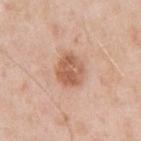Recorded during total-body skin imaging; not selected for excision or biopsy. A roughly 15 mm field-of-view crop from a total-body skin photograph. On the arm. A male subject aged 53–57. The lesion's longest dimension is about 4 mm. Captured under white-light illumination.